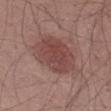imaging modality = ~15 mm tile from a whole-body skin photo
subject = male, aged around 55
lighting = white-light
diameter = about 6 mm
automated metrics = a shape eccentricity near 0.75 and two-axis asymmetry of about 0.1; a mean CIELAB color near L≈45 a*≈22 b*≈22, about 9 CIELAB-L* units darker than the surrounding skin, and a normalized border contrast of about 7; border irregularity of about 1.5 on a 0–10 scale, a color-variation rating of about 3/10, and peripheral color asymmetry of about 1; a classifier nevus-likeness of about 85/100 and lesion-presence confidence of about 100/100
location = the left thigh